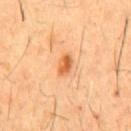No biopsy was performed on this lesion — it was imaged during a full skin examination and was not determined to be concerning. A male subject aged approximately 60. Measured at roughly 3 mm in maximum diameter. A 15 mm close-up extracted from a 3D total-body photography capture. Automated tile analysis of the lesion measured two-axis asymmetry of about 0.2. And it measured a lesion-to-skin contrast of about 8.5 (normalized; higher = more distinct). And it measured a border-irregularity index near 1.5/10, a within-lesion color-variation index near 4.5/10, and peripheral color asymmetry of about 1.5. On the chest. Captured under cross-polarized illumination.